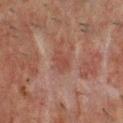follow-up — catalogued during a skin exam; not biopsied.